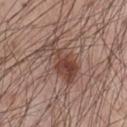notes = catalogued during a skin exam; not biopsied
automated lesion analysis = a lesion color around L≈43 a*≈20 b*≈24 in CIELAB, about 11 CIELAB-L* units darker than the surrounding skin, and a normalized border contrast of about 9; border irregularity of about 7.5 on a 0–10 scale and a color-variation rating of about 4/10
lighting = white-light
body site = the chest
patient = male, approximately 55 years of age
image source = total-body-photography crop, ~15 mm field of view